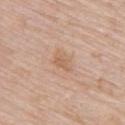Notes:
– notes: imaged on a skin check; not biopsied
– image: ~15 mm crop, total-body skin-cancer survey
– subject: female, aged 63–67
– lesion diameter: ≈2.5 mm
– anatomic site: the upper back
– TBP lesion metrics: a footprint of about 3 mm², an eccentricity of roughly 0.75, and two-axis asymmetry of about 0.35